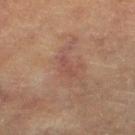Part of a total-body skin-imaging series; this lesion was reviewed on a skin check and was not flagged for biopsy. Measured at roughly 3 mm in maximum diameter. The lesion is on the left lower leg. A female subject about 80 years old. A 15 mm close-up tile from a total-body photography series done for melanoma screening. The tile uses cross-polarized illumination.